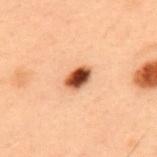• workup: catalogued during a skin exam; not biopsied